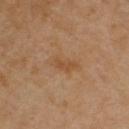Captured during whole-body skin photography for melanoma surveillance; the lesion was not biopsied. Automated image analysis of the tile measured an area of roughly 4 mm², an outline eccentricity of about 0.9 (0 = round, 1 = elongated), and a symmetry-axis asymmetry near 0.4. The software also gave a mean CIELAB color near L≈47 a*≈19 b*≈35, roughly 6 lightness units darker than nearby skin, and a normalized border contrast of about 6. And it measured lesion-presence confidence of about 100/100. A female subject, about 50 years old. On the arm. Cropped from a whole-body photographic skin survey; the tile spans about 15 mm. This is a cross-polarized tile.